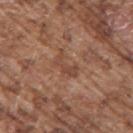Background:
A male subject approximately 75 years of age. A 15 mm close-up tile from a total-body photography series done for melanoma screening. The lesion's longest dimension is about 4 mm. From the right upper arm.